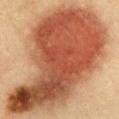follow-up: total-body-photography surveillance lesion; no biopsy
body site: the front of the torso
tile lighting: cross-polarized
imaging modality: total-body-photography crop, ~15 mm field of view
automated metrics: about 17 CIELAB-L* units darker than the surrounding skin and a normalized border contrast of about 11.5; a nevus-likeness score of about 100/100 and a lesion-detection confidence of about 100/100
subject: female, aged approximately 30